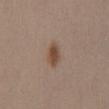biopsy status: no biopsy performed (imaged during a skin exam)
automated metrics: an automated nevus-likeness rating near 100 out of 100 and lesion-presence confidence of about 100/100
location: the mid back
image source: 15 mm crop, total-body photography
subject: female, aged approximately 30
diameter: about 3.5 mm